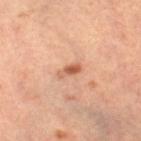• notes: imaged on a skin check; not biopsied
• site: the leg
• subject: female, in their mid-40s
• image source: ~15 mm crop, total-body skin-cancer survey
• illumination: cross-polarized illumination
• lesion size: ~3 mm (longest diameter)
• image-analysis metrics: a lesion–skin lightness drop of about 12 and a normalized border contrast of about 7.5; a nevus-likeness score of about 65/100 and a detector confidence of about 100 out of 100 that the crop contains a lesion